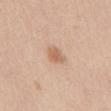Imaged during a routine full-body skin examination; the lesion was not biopsied and no histopathology is available. Imaged with white-light lighting. A female subject in their mid-30s. Measured at roughly 2.5 mm in maximum diameter. Cropped from a whole-body photographic skin survey; the tile spans about 15 mm. From the front of the torso.